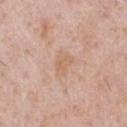Case summary:
- notes: no biopsy performed (imaged during a skin exam)
- subject: male, approximately 50 years of age
- image: 15 mm crop, total-body photography
- TBP lesion metrics: a symmetry-axis asymmetry near 0.25; an average lesion color of about L≈63 a*≈19 b*≈31 (CIELAB), roughly 6 lightness units darker than nearby skin, and a lesion-to-skin contrast of about 4.5 (normalized; higher = more distinct); a classifier nevus-likeness of about 0/100
- site: the chest